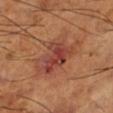biopsy status: total-body-photography surveillance lesion; no biopsy
subject: male, in their mid- to late 60s
image-analysis metrics: a lesion area of about 11 mm², a shape eccentricity near 0.7, and a shape-asymmetry score of about 0.4 (0 = symmetric)
body site: the leg
image: total-body-photography crop, ~15 mm field of view
illumination: cross-polarized illumination
lesion size: ≈5 mm